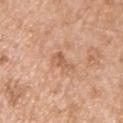{
  "biopsy_status": "not biopsied; imaged during a skin examination",
  "site": "left upper arm",
  "image": {
    "source": "total-body photography crop",
    "field_of_view_mm": 15
  },
  "automated_metrics": {
    "eccentricity": 0.8,
    "shape_asymmetry": 0.4,
    "border_irregularity_0_10": 4.0,
    "color_variation_0_10": 1.5,
    "peripheral_color_asymmetry": 0.5
  },
  "lighting": "white-light",
  "patient": {
    "sex": "male",
    "age_approx": 65
  }
}